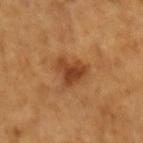workup = total-body-photography surveillance lesion; no biopsy | illumination = cross-polarized illumination | subject = female, approximately 55 years of age | lesion diameter = ≈3.5 mm | acquisition = 15 mm crop, total-body photography | anatomic site = the left forearm.